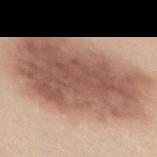Imaged during a routine full-body skin examination; the lesion was not biopsied and no histopathology is available.
Cropped from a whole-body photographic skin survey; the tile spans about 15 mm.
Automated image analysis of the tile measured a lesion area of about 80 mm², an outline eccentricity of about 0.85 (0 = round, 1 = elongated), and a shape-asymmetry score of about 0.1 (0 = symmetric).
The lesion's longest dimension is about 14 mm.
A female subject, aged 43–47.
The lesion is on the back.
Captured under white-light illumination.